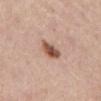The lesion was tiled from a total-body skin photograph and was not biopsied. Located on the front of the torso. A female patient, aged 58 to 62. The tile uses white-light illumination. An algorithmic analysis of the crop reported a mean CIELAB color near L≈53 a*≈21 b*≈29, a lesion–skin lightness drop of about 15, and a normalized border contrast of about 10.5. It also reported a border-irregularity index near 2/10 and radial color variation of about 1.5. Longest diameter approximately 3 mm. A close-up tile cropped from a whole-body skin photograph, about 15 mm across.